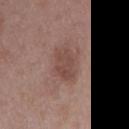| key | value |
|---|---|
| notes | imaged on a skin check; not biopsied |
| image-analysis metrics | a footprint of about 8.5 mm², an eccentricity of roughly 0.8, and a symmetry-axis asymmetry near 0.2; a lesion–skin lightness drop of about 8 and a normalized border contrast of about 6.5; a within-lesion color-variation index near 2.5/10 and a peripheral color-asymmetry measure near 1; a nevus-likeness score of about 5/100 and a lesion-detection confidence of about 100/100 |
| location | the right upper arm |
| subject | female, in their mid- to late 40s |
| tile lighting | white-light illumination |
| acquisition | 15 mm crop, total-body photography |